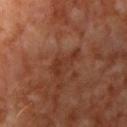  biopsy_status: not biopsied; imaged during a skin examination